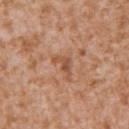Q: Is there a histopathology result?
A: catalogued during a skin exam; not biopsied
Q: Automated lesion metrics?
A: a footprint of about 3.5 mm², a shape eccentricity near 0.85, and two-axis asymmetry of about 0.55; an automated nevus-likeness rating near 0 out of 100 and a detector confidence of about 100 out of 100 that the crop contains a lesion
Q: Where on the body is the lesion?
A: the left upper arm
Q: What is the imaging modality?
A: 15 mm crop, total-body photography
Q: Who is the patient?
A: male, aged approximately 45
Q: What is the lesion's diameter?
A: ~3.5 mm (longest diameter)
Q: How was the tile lit?
A: white-light